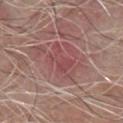Captured under white-light illumination.
The subject is a male aged around 80.
Cropped from a total-body skin-imaging series; the visible field is about 15 mm.
An algorithmic analysis of the crop reported an outline eccentricity of about 0.8 (0 = round, 1 = elongated) and a symmetry-axis asymmetry near 0.4. And it measured a lesion-detection confidence of about 65/100.
Longest diameter approximately 4.5 mm.
The lesion is on the chest.